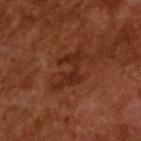* image source: ~15 mm crop, total-body skin-cancer survey
* lesion size: ~5 mm (longest diameter)
* subject: male, aged around 65
* lighting: cross-polarized illumination